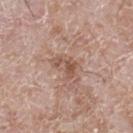The lesion was tiled from a total-body skin photograph and was not biopsied.
Measured at roughly 3 mm in maximum diameter.
Located on the right lower leg.
The tile uses white-light illumination.
A 15 mm crop from a total-body photograph taken for skin-cancer surveillance.
A male subject aged 58–62.